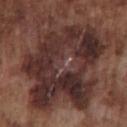| feature | finding |
|---|---|
| workup | imaged on a skin check; not biopsied |
| imaging modality | ~15 mm crop, total-body skin-cancer survey |
| body site | the chest |
| lighting | white-light illumination |
| lesion size | about 11.5 mm |
| patient | male, aged approximately 75 |
| automated lesion analysis | a lesion area of about 75 mm², a shape eccentricity near 0.55, and a symmetry-axis asymmetry near 0.3; a lesion color around L≈31 a*≈18 b*≈19 in CIELAB, roughly 11 lightness units darker than nearby skin, and a normalized lesion–skin contrast near 11 |